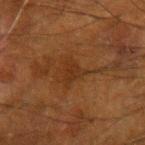location: the left forearm | patient: male, about 65 years old | lesion diameter: ~3 mm (longest diameter) | lighting: cross-polarized | image: total-body-photography crop, ~15 mm field of view | TBP lesion metrics: a footprint of about 5 mm², an outline eccentricity of about 0.4 (0 = round, 1 = elongated), and two-axis asymmetry of about 0.6; a mean CIELAB color near L≈25 a*≈18 b*≈28, roughly 5 lightness units darker than nearby skin, and a normalized border contrast of about 5.5.